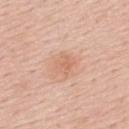The lesion was tiled from a total-body skin photograph and was not biopsied. Longest diameter approximately 2.5 mm. The lesion-visualizer software estimated a footprint of about 5 mm², a shape eccentricity near 0.6, and a symmetry-axis asymmetry near 0.2. The software also gave a lesion color around L≈65 a*≈23 b*≈32 in CIELAB and about 7 CIELAB-L* units darker than the surrounding skin. A roughly 15 mm field-of-view crop from a total-body skin photograph. Located on the upper back. A male subject aged around 55.